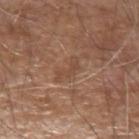Findings:
– biopsy status: imaged on a skin check; not biopsied
– acquisition: total-body-photography crop, ~15 mm field of view
– automated metrics: an area of roughly 3 mm², an eccentricity of roughly 0.95, and a symmetry-axis asymmetry near 0.65; a border-irregularity index near 7.5/10, internal color variation of about 0 on a 0–10 scale, and radial color variation of about 0
– location: the left forearm
– lighting: white-light illumination
– patient: male, aged approximately 80